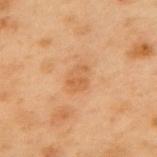Assessment: The lesion was tiled from a total-body skin photograph and was not biopsied. Clinical summary: On the upper back. Cropped from a whole-body photographic skin survey; the tile spans about 15 mm. A male subject, about 55 years old. The tile uses cross-polarized illumination. Approximately 3 mm at its widest.